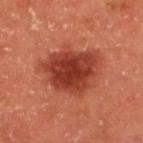The lesion was tiled from a total-body skin photograph and was not biopsied.
The lesion is on the upper back.
An algorithmic analysis of the crop reported an area of roughly 23 mm², an eccentricity of roughly 0.55, and a shape-asymmetry score of about 0.25 (0 = symmetric). It also reported a mean CIELAB color near L≈43 a*≈35 b*≈35 and a normalized lesion–skin contrast near 10. And it measured a nevus-likeness score of about 100/100 and a detector confidence of about 100 out of 100 that the crop contains a lesion.
A lesion tile, about 15 mm wide, cut from a 3D total-body photograph.
A female patient, aged approximately 50.
Approximately 6.5 mm at its widest.
This is a cross-polarized tile.